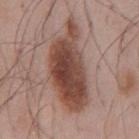  biopsy_status: not biopsied; imaged during a skin examination
  site: chest
  patient:
    sex: male
    age_approx: 55
  lighting: white-light
  image:
    source: total-body photography crop
    field_of_view_mm: 15
  lesion_size:
    long_diameter_mm_approx: 10.5